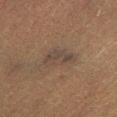Q: Was this lesion biopsied?
A: no biopsy performed (imaged during a skin exam)
Q: How was this image acquired?
A: total-body-photography crop, ~15 mm field of view
Q: Lesion location?
A: the leg
Q: What lighting was used for the tile?
A: cross-polarized
Q: What did automated image analysis measure?
A: a lesion area of about 7.5 mm² and a symmetry-axis asymmetry near 0.35; a classifier nevus-likeness of about 0/100 and a lesion-detection confidence of about 65/100
Q: How large is the lesion?
A: ~4 mm (longest diameter)
Q: Who is the patient?
A: male, aged approximately 40